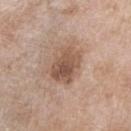Clinical impression: The lesion was tiled from a total-body skin photograph and was not biopsied. Context: A female subject aged around 75. The lesion is on the right forearm. A lesion tile, about 15 mm wide, cut from a 3D total-body photograph.